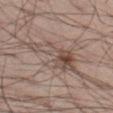Notes:
- workup — catalogued during a skin exam; not biopsied
- tile lighting — white-light illumination
- subject — male, approximately 55 years of age
- automated lesion analysis — a lesion area of about 16 mm², an eccentricity of roughly 0.9, and a symmetry-axis asymmetry near 0.5; an average lesion color of about L≈50 a*≈14 b*≈22 (CIELAB), a lesion–skin lightness drop of about 9, and a normalized lesion–skin contrast near 6.5; border irregularity of about 8 on a 0–10 scale, internal color variation of about 9 on a 0–10 scale, and a peripheral color-asymmetry measure near 4
- anatomic site — the left thigh
- image source — ~15 mm crop, total-body skin-cancer survey